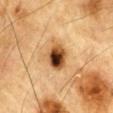biopsy_status: not biopsied; imaged during a skin examination
lesion_size:
  long_diameter_mm_approx: 3.5
lighting: cross-polarized
site: chest
patient:
  sex: male
  age_approx: 85+
image:
  source: total-body photography crop
  field_of_view_mm: 15